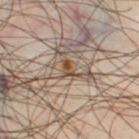Captured during whole-body skin photography for melanoma surveillance; the lesion was not biopsied.